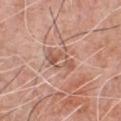Assessment:
Part of a total-body skin-imaging series; this lesion was reviewed on a skin check and was not flagged for biopsy.
Background:
Imaged with white-light lighting. A region of skin cropped from a whole-body photographic capture, roughly 15 mm wide. From the chest. The lesion-visualizer software estimated a border-irregularity rating of about 3/10 and internal color variation of about 7 on a 0–10 scale. It also reported a nevus-likeness score of about 0/100. The lesion's longest dimension is about 4 mm. A male patient aged approximately 60.